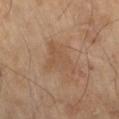Clinical impression:
Part of a total-body skin-imaging series; this lesion was reviewed on a skin check and was not flagged for biopsy.
Background:
On the right thigh. The tile uses cross-polarized illumination. The lesion's longest dimension is about 6 mm. A male subject, aged approximately 70. A roughly 15 mm field-of-view crop from a total-body skin photograph. The total-body-photography lesion software estimated a mean CIELAB color near L≈49 a*≈17 b*≈30, roughly 6 lightness units darker than nearby skin, and a normalized border contrast of about 4.5. The software also gave a classifier nevus-likeness of about 0/100 and a lesion-detection confidence of about 100/100.